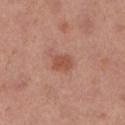<record>
<biopsy_status>not biopsied; imaged during a skin examination</biopsy_status>
<automated_metrics>
  <cielab_L>51</cielab_L>
  <cielab_a>25</cielab_a>
  <cielab_b>30</cielab_b>
  <vs_skin_darker_L>9.0</vs_skin_darker_L>
  <vs_skin_contrast_norm>7.0</vs_skin_contrast_norm>
</automated_metrics>
<image>
  <source>total-body photography crop</source>
  <field_of_view_mm>15</field_of_view_mm>
</image>
<lighting>white-light</lighting>
<site>right lower leg</site>
<lesion_size>
  <long_diameter_mm_approx>2.5</long_diameter_mm_approx>
</lesion_size>
<patient>
  <sex>female</sex>
  <age_approx>40</age_approx>
</patient>
</record>